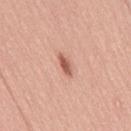Recorded during total-body skin imaging; not selected for excision or biopsy.
Imaged with white-light lighting.
The lesion's longest dimension is about 3 mm.
A male subject, in their 50s.
A roughly 15 mm field-of-view crop from a total-body skin photograph.
Automated tile analysis of the lesion measured a within-lesion color-variation index near 1/10 and radial color variation of about 0.5.
Located on the leg.